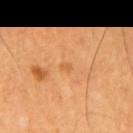Impression:
Imaged during a routine full-body skin examination; the lesion was not biopsied and no histopathology is available.
Background:
On the mid back. The lesion's longest dimension is about 1.5 mm. A 15 mm crop from a total-body photograph taken for skin-cancer surveillance. The patient is a male approximately 60 years of age. Automated image analysis of the tile measured a lesion area of about 1 mm² and two-axis asymmetry of about 0.35. The software also gave a nevus-likeness score of about 0/100 and a detector confidence of about 100 out of 100 that the crop contains a lesion.